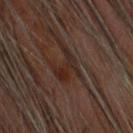{
  "biopsy_status": "not biopsied; imaged during a skin examination",
  "patient": {
    "sex": "male",
    "age_approx": 65
  },
  "image": {
    "source": "total-body photography crop",
    "field_of_view_mm": 15
  },
  "site": "head or neck"
}